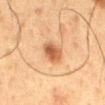– biopsy status — imaged on a skin check; not biopsied
– lighting — cross-polarized
– subject — male, in their 60s
– lesion size — ~3 mm (longest diameter)
– anatomic site — the mid back
– image source — ~15 mm crop, total-body skin-cancer survey